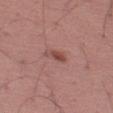Clinical impression: Captured during whole-body skin photography for melanoma surveillance; the lesion was not biopsied. Acquisition and patient details: The lesion's longest dimension is about 3 mm. A region of skin cropped from a whole-body photographic capture, roughly 15 mm wide. The lesion is located on the right thigh. A male patient, aged 43 to 47. Captured under white-light illumination. An algorithmic analysis of the crop reported a within-lesion color-variation index near 1/10 and radial color variation of about 0.5. And it measured an automated nevus-likeness rating near 85 out of 100.